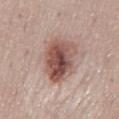Impression: Captured during whole-body skin photography for melanoma surveillance; the lesion was not biopsied. Background: A close-up tile cropped from a whole-body skin photograph, about 15 mm across. Automated image analysis of the tile measured an area of roughly 20 mm², an outline eccentricity of about 0.7 (0 = round, 1 = elongated), and a symmetry-axis asymmetry near 0.2. And it measured a lesion color around L≈52 a*≈20 b*≈23 in CIELAB, roughly 15 lightness units darker than nearby skin, and a normalized lesion–skin contrast near 10. The lesion's longest dimension is about 6 mm. From the mid back. The tile uses white-light illumination. A female subject aged around 50.